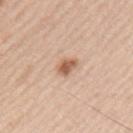Clinical impression: This lesion was catalogued during total-body skin photography and was not selected for biopsy. Clinical summary: An algorithmic analysis of the crop reported a lesion color around L≈60 a*≈21 b*≈32 in CIELAB, a lesion–skin lightness drop of about 13, and a normalized border contrast of about 8.5. A male patient roughly 45 years of age. Longest diameter approximately 3 mm. On the back. Captured under white-light illumination. A 15 mm close-up extracted from a 3D total-body photography capture.